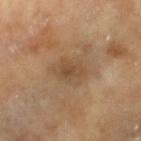  biopsy_status: not biopsied; imaged during a skin examination